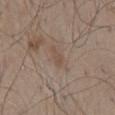The lesion was photographed on a routine skin check and not biopsied; there is no pathology result. The tile uses white-light illumination. The lesion is on the mid back. A male subject, in their mid- to late 50s. Automated tile analysis of the lesion measured a lesion area of about 3 mm² and an eccentricity of roughly 0.9. The analysis additionally found a border-irregularity index near 3/10, a within-lesion color-variation index near 1/10, and peripheral color asymmetry of about 0. Measured at roughly 2.5 mm in maximum diameter. A lesion tile, about 15 mm wide, cut from a 3D total-body photograph.